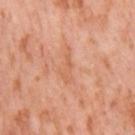| feature | finding |
|---|---|
| biopsy status | catalogued during a skin exam; not biopsied |
| site | the right thigh |
| subject | female, about 55 years old |
| illumination | cross-polarized illumination |
| image-analysis metrics | a mean CIELAB color near L≈63 a*≈26 b*≈36 and a lesion–skin lightness drop of about 6; a border-irregularity index near 7/10, a color-variation rating of about 0.5/10, and radial color variation of about 0; a detector confidence of about 90 out of 100 that the crop contains a lesion |
| diameter | ~4 mm (longest diameter) |
| acquisition | ~15 mm tile from a whole-body skin photo |